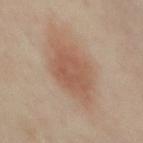Part of a total-body skin-imaging series; this lesion was reviewed on a skin check and was not flagged for biopsy.
The recorded lesion diameter is about 7 mm.
This is a cross-polarized tile.
A male patient, aged around 50.
Located on the chest.
This image is a 15 mm lesion crop taken from a total-body photograph.
Automated image analysis of the tile measured an area of roughly 21 mm², an eccentricity of roughly 0.8, and a symmetry-axis asymmetry near 0.2. And it measured a nevus-likeness score of about 100/100.